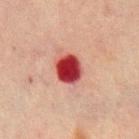The lesion was photographed on a routine skin check and not biopsied; there is no pathology result. Longest diameter approximately 3.5 mm. Cropped from a whole-body photographic skin survey; the tile spans about 15 mm. A male subject approximately 70 years of age. This is a cross-polarized tile. The lesion is located on the back.